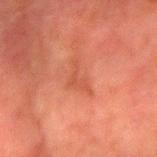| feature | finding |
|---|---|
| imaging modality | total-body-photography crop, ~15 mm field of view |
| tile lighting | cross-polarized illumination |
| automated lesion analysis | a lesion area of about 4.5 mm², a shape eccentricity near 0.9, and two-axis asymmetry of about 0.7; a lesion color around L≈42 a*≈27 b*≈30 in CIELAB, a lesion–skin lightness drop of about 5, and a normalized border contrast of about 4.5; a nevus-likeness score of about 0/100 and a detector confidence of about 95 out of 100 that the crop contains a lesion |
| location | the arm |
| diameter | about 4 mm |
| subject | male, aged 78 to 82 |